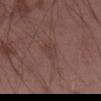Impression:
This lesion was catalogued during total-body skin photography and was not selected for biopsy.
Acquisition and patient details:
The patient is a male about 50 years old. Imaged with white-light lighting. On the left thigh. Longest diameter approximately 2.5 mm. A close-up tile cropped from a whole-body skin photograph, about 15 mm across. The lesion-visualizer software estimated an outline eccentricity of about 0.8 (0 = round, 1 = elongated) and a symmetry-axis asymmetry near 0.3. And it measured an average lesion color of about L≈39 a*≈19 b*≈21 (CIELAB), roughly 5 lightness units darker than nearby skin, and a lesion-to-skin contrast of about 5 (normalized; higher = more distinct). The analysis additionally found a classifier nevus-likeness of about 0/100 and lesion-presence confidence of about 100/100.